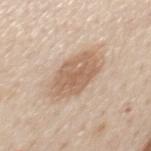  biopsy_status: not biopsied; imaged during a skin examination
  image:
    source: total-body photography crop
    field_of_view_mm: 15
  patient:
    sex: male
    age_approx: 60
  site: mid back